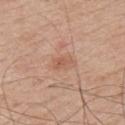The lesion was tiled from a total-body skin photograph and was not biopsied.
A male patient, aged approximately 70.
Cropped from a total-body skin-imaging series; the visible field is about 15 mm.
From the upper back.
The lesion-visualizer software estimated a lesion area of about 3 mm² and an eccentricity of roughly 0.85. And it measured a mean CIELAB color near L≈57 a*≈22 b*≈31 and a normalized lesion–skin contrast near 5.5. The analysis additionally found border irregularity of about 3 on a 0–10 scale and a within-lesion color-variation index near 1/10. The analysis additionally found an automated nevus-likeness rating near 0 out of 100 and lesion-presence confidence of about 100/100.
Imaged with white-light lighting.
The recorded lesion diameter is about 2.5 mm.